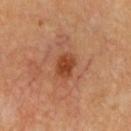Impression:
Imaged during a routine full-body skin examination; the lesion was not biopsied and no histopathology is available.
Background:
Longest diameter approximately 3 mm. The lesion is located on the chest. This is a cross-polarized tile. A 15 mm crop from a total-body photograph taken for skin-cancer surveillance. A male subject approximately 60 years of age.